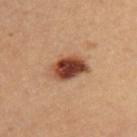{
  "biopsy_status": "not biopsied; imaged during a skin examination",
  "lighting": "cross-polarized",
  "site": "upper back",
  "lesion_size": {
    "long_diameter_mm_approx": 5.0
  },
  "image": {
    "source": "total-body photography crop",
    "field_of_view_mm": 15
  },
  "patient": {
    "sex": "female",
    "age_approx": 45
  },
  "automated_metrics": {
    "cielab_L": 45,
    "cielab_a": 24,
    "cielab_b": 31,
    "vs_skin_darker_L": 18.0,
    "vs_skin_contrast_norm": 13.0,
    "border_irregularity_0_10": 2.0,
    "color_variation_0_10": 8.5,
    "peripheral_color_asymmetry": 2.5,
    "nevus_likeness_0_100": 100,
    "lesion_detection_confidence_0_100": 100
  }
}